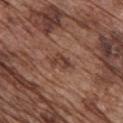Q: Is there a histopathology result?
A: no biopsy performed (imaged during a skin exam)
Q: What lighting was used for the tile?
A: white-light illumination
Q: Where on the body is the lesion?
A: the chest
Q: What kind of image is this?
A: ~15 mm crop, total-body skin-cancer survey
Q: Lesion size?
A: about 2.5 mm
Q: Who is the patient?
A: male, in their mid- to late 70s
Q: What did automated image analysis measure?
A: a footprint of about 3.5 mm², an eccentricity of roughly 0.8, and a shape-asymmetry score of about 0.45 (0 = symmetric); a nevus-likeness score of about 0/100 and lesion-presence confidence of about 100/100